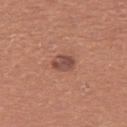Recorded during total-body skin imaging; not selected for excision or biopsy. On the left thigh. The tile uses white-light illumination. A region of skin cropped from a whole-body photographic capture, roughly 15 mm wide. A female subject, aged 38 to 42. Automated tile analysis of the lesion measured a mean CIELAB color near L≈48 a*≈23 b*≈25, about 11 CIELAB-L* units darker than the surrounding skin, and a normalized lesion–skin contrast near 8.5. The software also gave a border-irregularity rating of about 1.5/10 and a within-lesion color-variation index near 3/10.